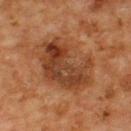• acquisition — ~15 mm crop, total-body skin-cancer survey
• subject — male, approximately 65 years of age
• body site — the back
• lesion size — ≈8 mm
• tile lighting — cross-polarized illumination